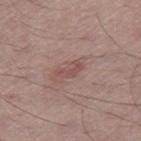<case>
<biopsy_status>not biopsied; imaged during a skin examination</biopsy_status>
<lesion_size>
  <long_diameter_mm_approx>3.0</long_diameter_mm_approx>
</lesion_size>
<site>right thigh</site>
<lighting>white-light</lighting>
<image>
  <source>total-body photography crop</source>
  <field_of_view_mm>15</field_of_view_mm>
</image>
<patient>
  <sex>male</sex>
  <age_approx>50</age_approx>
</patient>
</case>